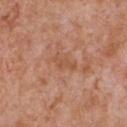<lesion>
<biopsy_status>not biopsied; imaged during a skin examination</biopsy_status>
<site>chest</site>
<image>
  <source>total-body photography crop</source>
  <field_of_view_mm>15</field_of_view_mm>
</image>
<automated_metrics>
  <area_mm2_approx>2.5</area_mm2_approx>
  <eccentricity>0.9</eccentricity>
  <cielab_L>52</cielab_L>
  <cielab_a>24</cielab_a>
  <cielab_b>34</cielab_b>
  <vs_skin_darker_L>6.0</vs_skin_darker_L>
  <vs_skin_contrast_norm>5.5</vs_skin_contrast_norm>
  <border_irregularity_0_10>5.5</border_irregularity_0_10>
  <color_variation_0_10>0.0</color_variation_0_10>
  <nevus_likeness_0_100>0</nevus_likeness_0_100>
  <lesion_detection_confidence_0_100>100</lesion_detection_confidence_0_100>
</automated_metrics>
<patient>
  <sex>male</sex>
  <age_approx>65</age_approx>
</patient>
</lesion>